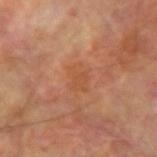  biopsy_status: not biopsied; imaged during a skin examination
  image:
    source: total-body photography crop
    field_of_view_mm: 15
  patient:
    sex: male
    age_approx: 70
  site: left forearm